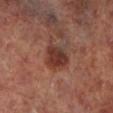Imaged with cross-polarized lighting. A 15 mm close-up tile from a total-body photography series done for melanoma screening. Measured at roughly 3.5 mm in maximum diameter. From the left lower leg. An algorithmic analysis of the crop reported a lesion area of about 8 mm², an eccentricity of roughly 0.5, and a symmetry-axis asymmetry near 0.25. It also reported an average lesion color of about L≈35 a*≈23 b*≈25 (CIELAB), roughly 10 lightness units darker than nearby skin, and a lesion-to-skin contrast of about 9 (normalized; higher = more distinct). The analysis additionally found border irregularity of about 2.5 on a 0–10 scale and internal color variation of about 4.5 on a 0–10 scale. A male subject, aged 63 to 67.